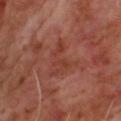Acquisition and patient details:
The subject is a male aged around 65. Longest diameter approximately 4.5 mm. The lesion is on the upper back. This image is a 15 mm lesion crop taken from a total-body photograph. Imaged with cross-polarized lighting.